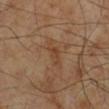A male patient, in their 70s. A 15 mm close-up tile from a total-body photography series done for melanoma screening. An algorithmic analysis of the crop reported an average lesion color of about L≈41 a*≈19 b*≈31 (CIELAB) and roughly 6 lightness units darker than nearby skin. This is a cross-polarized tile. The lesion is located on the right lower leg. Approximately 4 mm at its widest.